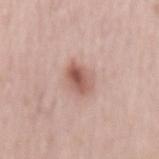No biopsy was performed on this lesion — it was imaged during a full skin examination and was not determined to be concerning. A male patient, aged 63–67. A region of skin cropped from a whole-body photographic capture, roughly 15 mm wide. The lesion is located on the lower back.